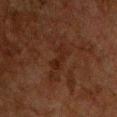{
  "biopsy_status": "not biopsied; imaged during a skin examination",
  "automated_metrics": {
    "area_mm2_approx": 3.5,
    "eccentricity": 0.9,
    "shape_asymmetry": 0.35,
    "nevus_likeness_0_100": 0,
    "lesion_detection_confidence_0_100": 100
  },
  "patient": {
    "sex": "male",
    "age_approx": 60
  },
  "image": {
    "source": "total-body photography crop",
    "field_of_view_mm": 15
  },
  "lighting": "cross-polarized",
  "lesion_size": {
    "long_diameter_mm_approx": 3.0
  },
  "site": "chest"
}